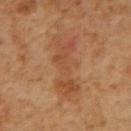Clinical impression:
This lesion was catalogued during total-body skin photography and was not selected for biopsy.
Background:
Longest diameter approximately 8 mm. A 15 mm close-up extracted from a 3D total-body photography capture. Located on the back. This is a cross-polarized tile. A male patient about 60 years old.